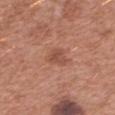This lesion was catalogued during total-body skin photography and was not selected for biopsy. Approximately 3.5 mm at its widest. Captured under white-light illumination. Cropped from a total-body skin-imaging series; the visible field is about 15 mm. Located on the left forearm. A female subject approximately 40 years of age. An algorithmic analysis of the crop reported a lesion area of about 6 mm² and a shape-asymmetry score of about 0.35 (0 = symmetric). The analysis additionally found an average lesion color of about L≈51 a*≈24 b*≈29 (CIELAB) and a normalized lesion–skin contrast near 5.5. The software also gave border irregularity of about 3.5 on a 0–10 scale, a within-lesion color-variation index near 2.5/10, and peripheral color asymmetry of about 1. It also reported an automated nevus-likeness rating near 5 out of 100 and a detector confidence of about 100 out of 100 that the crop contains a lesion.